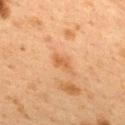biopsy status: catalogued during a skin exam; not biopsied
lesion diameter: ≈2.5 mm
image source: 15 mm crop, total-body photography
image-analysis metrics: an automated nevus-likeness rating near 10 out of 100 and a detector confidence of about 100 out of 100 that the crop contains a lesion
body site: the upper back
subject: female, roughly 40 years of age